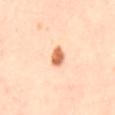automated lesion analysis = a border-irregularity index near 2/10, internal color variation of about 3.5 on a 0–10 scale, and peripheral color asymmetry of about 1; lesion diameter = about 2.5 mm; lighting = cross-polarized illumination; image source = total-body-photography crop, ~15 mm field of view; anatomic site = the right thigh; patient = female, approximately 40 years of age.